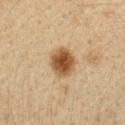The recorded lesion diameter is about 3.5 mm.
A close-up tile cropped from a whole-body skin photograph, about 15 mm across.
Located on the left forearm.
Imaged with cross-polarized lighting.
The patient is a female about 40 years old.
The total-body-photography lesion software estimated a shape eccentricity near 0.5 and a symmetry-axis asymmetry near 0.15. The software also gave a mean CIELAB color near L≈42 a*≈15 b*≈31, a lesion–skin lightness drop of about 14, and a normalized lesion–skin contrast near 11.